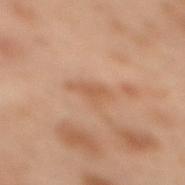follow-up — total-body-photography surveillance lesion; no biopsy | lesion size — ~3.5 mm (longest diameter) | lighting — cross-polarized illumination | image source — total-body-photography crop, ~15 mm field of view | subject — female, approximately 55 years of age | body site — the upper back.